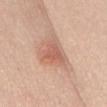workup = total-body-photography surveillance lesion; no biopsy.